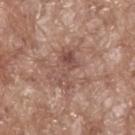Case summary:
* patient: male, approximately 70 years of age
* tile lighting: white-light
* acquisition: ~15 mm tile from a whole-body skin photo
* anatomic site: the back
* lesion size: about 5 mm
* TBP lesion metrics: an area of roughly 7 mm², a shape eccentricity near 0.9, and two-axis asymmetry of about 0.5; an average lesion color of about L≈49 a*≈20 b*≈24 (CIELAB)